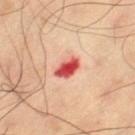Impression: This lesion was catalogued during total-body skin photography and was not selected for biopsy. Background: A 15 mm close-up extracted from a 3D total-body photography capture. Measured at roughly 3 mm in maximum diameter. On the left thigh. The patient is a male aged approximately 60. Imaged with cross-polarized lighting.